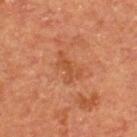biopsy_status: not biopsied; imaged during a skin examination
site: upper back
patient:
  sex: male
  age_approx: 55
image:
  source: total-body photography crop
  field_of_view_mm: 15
automated_metrics:
  area_mm2_approx: 6.0
  eccentricity: 0.85
  shape_asymmetry: 0.45
  border_irregularity_0_10: 5.0
  color_variation_0_10: 2.0
  peripheral_color_asymmetry: 0.5
lesion_size:
  long_diameter_mm_approx: 4.0